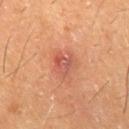The lesion was photographed on a routine skin check and not biopsied; there is no pathology result. Captured under cross-polarized illumination. Cropped from a whole-body photographic skin survey; the tile spans about 15 mm. Approximately 3 mm at its widest. An algorithmic analysis of the crop reported an area of roughly 6 mm², an outline eccentricity of about 0.1 (0 = round, 1 = elongated), and a symmetry-axis asymmetry near 0.3. It also reported an average lesion color of about L≈52 a*≈28 b*≈31 (CIELAB). The software also gave border irregularity of about 3 on a 0–10 scale and peripheral color asymmetry of about 2.5. It also reported a classifier nevus-likeness of about 0/100 and a lesion-detection confidence of about 100/100. The patient is a male in their 60s. On the right thigh.